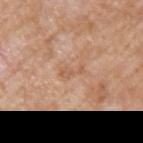Clinical impression:
The lesion was tiled from a total-body skin photograph and was not biopsied.
Image and clinical context:
A region of skin cropped from a whole-body photographic capture, roughly 15 mm wide. A male patient about 60 years old. Imaged with white-light lighting. Longest diameter approximately 3 mm. The lesion is located on the left upper arm.